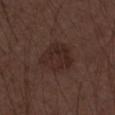Findings:
- workup: imaged on a skin check; not biopsied
- acquisition: ~15 mm tile from a whole-body skin photo
- size: ≈4.5 mm
- tile lighting: white-light
- subject: male, aged 48 to 52